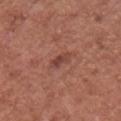Q: Was this lesion biopsied?
A: no biopsy performed (imaged during a skin exam)
Q: Illumination type?
A: white-light illumination
Q: Where on the body is the lesion?
A: the chest
Q: What is the imaging modality?
A: ~15 mm crop, total-body skin-cancer survey
Q: Patient demographics?
A: male, about 75 years old
Q: How large is the lesion?
A: about 2.5 mm